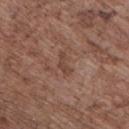Image and clinical context: The patient is a male approximately 70 years of age. This is a white-light tile. From the chest. The recorded lesion diameter is about 2.5 mm. A 15 mm close-up tile from a total-body photography series done for melanoma screening. Automated image analysis of the tile measured an area of roughly 2.5 mm², an eccentricity of roughly 0.9, and a shape-asymmetry score of about 0.55 (0 = symmetric). It also reported an average lesion color of about L≈43 a*≈19 b*≈26 (CIELAB), about 7 CIELAB-L* units darker than the surrounding skin, and a lesion-to-skin contrast of about 6 (normalized; higher = more distinct). And it measured a border-irregularity rating of about 6/10, internal color variation of about 0 on a 0–10 scale, and radial color variation of about 0.